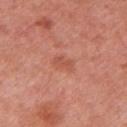Assessment: Part of a total-body skin-imaging series; this lesion was reviewed on a skin check and was not flagged for biopsy. Context: A female patient, aged around 50. Cropped from a whole-body photographic skin survey; the tile spans about 15 mm. On the left upper arm.